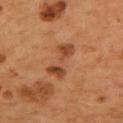workup=no biopsy performed (imaged during a skin exam)
anatomic site=the mid back
tile lighting=cross-polarized
patient=male, in their mid-50s
size=~4.5 mm (longest diameter)
imaging modality=~15 mm crop, total-body skin-cancer survey